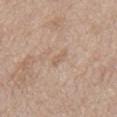{"biopsy_status": "not biopsied; imaged during a skin examination", "site": "front of the torso", "patient": {"sex": "male", "age_approx": 80}, "image": {"source": "total-body photography crop", "field_of_view_mm": 15}}